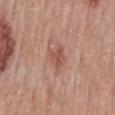Background:
Cropped from a whole-body photographic skin survey; the tile spans about 15 mm. The lesion's longest dimension is about 3 mm. The patient is a male about 50 years old. Imaged with white-light lighting. Located on the mid back.